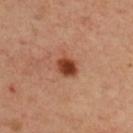notes — no biopsy performed (imaged during a skin exam)
patient — male, in their mid-50s
tile lighting — cross-polarized
body site — the upper back
TBP lesion metrics — an area of roughly 5 mm², a shape eccentricity near 0.55, and a symmetry-axis asymmetry near 0.2; a border-irregularity rating of about 1.5/10, a within-lesion color-variation index near 4/10, and peripheral color asymmetry of about 1.5; a classifier nevus-likeness of about 100/100 and a lesion-detection confidence of about 100/100
image — ~15 mm crop, total-body skin-cancer survey
lesion diameter — ≈2.5 mm